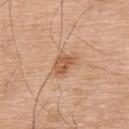<case>
<biopsy_status>not biopsied; imaged during a skin examination</biopsy_status>
<image>
  <source>total-body photography crop</source>
  <field_of_view_mm>15</field_of_view_mm>
</image>
<patient>
  <sex>male</sex>
  <age_approx>75</age_approx>
</patient>
<lighting>white-light</lighting>
<site>upper back</site>
</case>